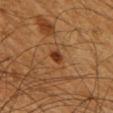Clinical impression:
Recorded during total-body skin imaging; not selected for excision or biopsy.
Clinical summary:
The tile uses cross-polarized illumination. The lesion is on the right upper arm. The total-body-photography lesion software estimated roughly 11 lightness units darker than nearby skin. The software also gave a border-irregularity index near 2/10 and a peripheral color-asymmetry measure near 0.5. It also reported an automated nevus-likeness rating near 95 out of 100. The lesion's longest dimension is about 2 mm. A male patient roughly 60 years of age. A close-up tile cropped from a whole-body skin photograph, about 15 mm across.